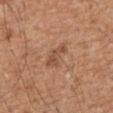Findings:
- follow-up · catalogued during a skin exam; not biopsied
- subject · male, about 65 years old
- location · the abdomen
- image source · ~15 mm tile from a whole-body skin photo